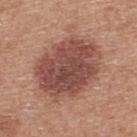This lesion was catalogued during total-body skin photography and was not selected for biopsy. On the upper back. A 15 mm close-up extracted from a 3D total-body photography capture. An algorithmic analysis of the crop reported an area of roughly 36 mm², an eccentricity of roughly 0.65, and a symmetry-axis asymmetry near 0.1. And it measured border irregularity of about 1.5 on a 0–10 scale, a color-variation rating of about 5/10, and a peripheral color-asymmetry measure near 1.5. The analysis additionally found a nevus-likeness score of about 50/100 and lesion-presence confidence of about 100/100. A male subject aged 28–32. The lesion's longest dimension is about 8 mm. Captured under white-light illumination.